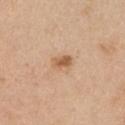Impression:
Imaged during a routine full-body skin examination; the lesion was not biopsied and no histopathology is available.
Clinical summary:
This is a white-light tile. On the front of the torso. This image is a 15 mm lesion crop taken from a total-body photograph. The lesion's longest dimension is about 2.5 mm. A female patient roughly 45 years of age.